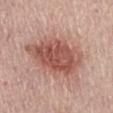notes = imaged on a skin check; not biopsied | illumination = white-light illumination | site = the abdomen | lesion diameter = about 7.5 mm | image source = total-body-photography crop, ~15 mm field of view | subject = female, about 65 years old.